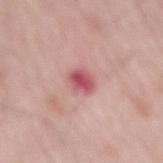{"biopsy_status": "not biopsied; imaged during a skin examination", "image": {"source": "total-body photography crop", "field_of_view_mm": 15}, "lighting": "white-light", "automated_metrics": {"area_mm2_approx": 4.5, "eccentricity": 0.65, "cielab_L": 55, "cielab_a": 33, "cielab_b": 20, "vs_skin_darker_L": 14.0}, "site": "back", "patient": {"sex": "male", "age_approx": 65}, "lesion_size": {"long_diameter_mm_approx": 3.0}}